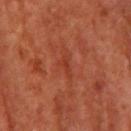  biopsy_status: not biopsied; imaged during a skin examination
  image:
    source: total-body photography crop
    field_of_view_mm: 15
  site: upper back
  lesion_size:
    long_diameter_mm_approx: 3.0
  patient:
    sex: male
    age_approx: 70
  lighting: cross-polarized